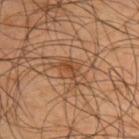Part of a total-body skin-imaging series; this lesion was reviewed on a skin check and was not flagged for biopsy. The lesion-visualizer software estimated an area of roughly 4.5 mm², a shape eccentricity near 0.6, and a symmetry-axis asymmetry near 0.15. It also reported an average lesion color of about L≈44 a*≈21 b*≈34 (CIELAB), a lesion–skin lightness drop of about 7, and a normalized border contrast of about 6. A male patient, roughly 45 years of age. A 15 mm close-up extracted from a 3D total-body photography capture. Located on the back.